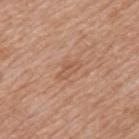Q: What did automated image analysis measure?
A: an average lesion color of about L≈56 a*≈21 b*≈32 (CIELAB), about 6 CIELAB-L* units darker than the surrounding skin, and a normalized border contrast of about 4.5; a border-irregularity rating of about 4.5/10 and peripheral color asymmetry of about 1
Q: What is the lesion's diameter?
A: ≈3 mm
Q: What kind of image is this?
A: 15 mm crop, total-body photography
Q: Illumination type?
A: white-light
Q: What are the patient's age and sex?
A: male, in their 60s
Q: Lesion location?
A: the back